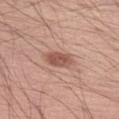follow-up — no biopsy performed (imaged during a skin exam) | illumination — white-light illumination | image source — 15 mm crop, total-body photography | subject — male, about 55 years old | body site — the left thigh.